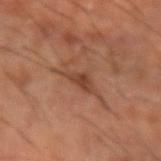{
  "biopsy_status": "not biopsied; imaged during a skin examination",
  "patient": {
    "sex": "male",
    "age_approx": 60
  },
  "image": {
    "source": "total-body photography crop",
    "field_of_view_mm": 15
  },
  "lesion_size": {
    "long_diameter_mm_approx": 6.0
  },
  "site": "right forearm"
}